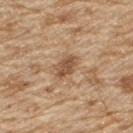workup: catalogued during a skin exam; not biopsied | body site: the upper back | illumination: white-light illumination | acquisition: total-body-photography crop, ~15 mm field of view | image-analysis metrics: a footprint of about 5 mm² and a shape eccentricity near 0.75; an average lesion color of about L≈52 a*≈19 b*≈33 (CIELAB) and a normalized border contrast of about 8; border irregularity of about 2.5 on a 0–10 scale, internal color variation of about 2 on a 0–10 scale, and peripheral color asymmetry of about 1; a lesion-detection confidence of about 100/100 | subject: male, roughly 70 years of age.